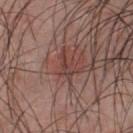| feature | finding |
|---|---|
| biopsy status | no biopsy performed (imaged during a skin exam) |
| illumination | white-light illumination |
| automated metrics | a border-irregularity rating of about 5/10, a within-lesion color-variation index near 0/10, and radial color variation of about 0 |
| site | the chest |
| acquisition | ~15 mm tile from a whole-body skin photo |
| patient | male, approximately 25 years of age |